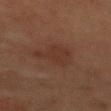Clinical impression: Imaged during a routine full-body skin examination; the lesion was not biopsied and no histopathology is available. Clinical summary: The subject is a male roughly 60 years of age. A 15 mm crop from a total-body photograph taken for skin-cancer surveillance. The lesion is on the chest. Automated image analysis of the tile measured a lesion color around L≈30 a*≈18 b*≈23 in CIELAB and a normalized lesion–skin contrast near 5. The analysis additionally found border irregularity of about 4 on a 0–10 scale and peripheral color asymmetry of about 0.5.